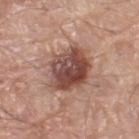| field | value |
|---|---|
| illumination | white-light illumination |
| acquisition | ~15 mm crop, total-body skin-cancer survey |
| anatomic site | the left thigh |
| patient | male, roughly 80 years of age |
| size | ≈5.5 mm |